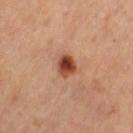Recorded during total-body skin imaging; not selected for excision or biopsy. Captured under cross-polarized illumination. The lesion is on the left thigh. A female patient about 65 years old. Approximately 2.5 mm at its widest. A lesion tile, about 15 mm wide, cut from a 3D total-body photograph.